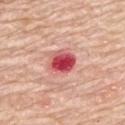| feature | finding |
|---|---|
| tile lighting | white-light illumination |
| diameter | ≈3.5 mm |
| automated metrics | an area of roughly 8 mm², an eccentricity of roughly 0.6, and a symmetry-axis asymmetry near 0.15; a lesion color around L≈53 a*≈41 b*≈25 in CIELAB and a lesion-to-skin contrast of about 12 (normalized; higher = more distinct); radial color variation of about 2.5 |
| location | the upper back |
| image source | total-body-photography crop, ~15 mm field of view |
| subject | female, aged 63 to 67 |